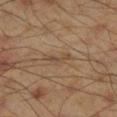Q: Was a biopsy performed?
A: catalogued during a skin exam; not biopsied
Q: What are the patient's age and sex?
A: male, roughly 45 years of age
Q: What is the anatomic site?
A: the leg
Q: What is the lesion's diameter?
A: ~3.5 mm (longest diameter)
Q: Illumination type?
A: cross-polarized illumination
Q: How was this image acquired?
A: total-body-photography crop, ~15 mm field of view